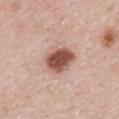Q: Was a biopsy performed?
A: imaged on a skin check; not biopsied
Q: How was the tile lit?
A: white-light
Q: How was this image acquired?
A: ~15 mm tile from a whole-body skin photo
Q: What is the lesion's diameter?
A: about 4 mm
Q: Automated lesion metrics?
A: an average lesion color of about L≈53 a*≈23 b*≈27 (CIELAB) and about 18 CIELAB-L* units darker than the surrounding skin
Q: What is the anatomic site?
A: the mid back
Q: Patient demographics?
A: female, aged 28 to 32